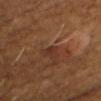Findings:
- follow-up: no biopsy performed (imaged during a skin exam)
- lesion size: ~1.5 mm (longest diameter)
- acquisition: total-body-photography crop, ~15 mm field of view
- patient: male, in their mid-40s
- site: the chest
- lighting: cross-polarized illumination